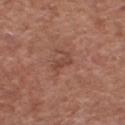{
  "biopsy_status": "not biopsied; imaged during a skin examination",
  "lesion_size": {
    "long_diameter_mm_approx": 3.0
  },
  "site": "chest",
  "lighting": "white-light",
  "patient": {
    "sex": "male",
    "age_approx": 75
  },
  "image": {
    "source": "total-body photography crop",
    "field_of_view_mm": 15
  }
}